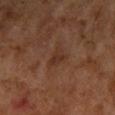Findings:
– workup: imaged on a skin check; not biopsied